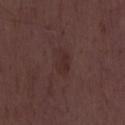Clinical impression:
Captured during whole-body skin photography for melanoma surveillance; the lesion was not biopsied.
Background:
Cropped from a total-body skin-imaging series; the visible field is about 15 mm. Automated image analysis of the tile measured an area of roughly 5 mm², an outline eccentricity of about 0.85 (0 = round, 1 = elongated), and a shape-asymmetry score of about 0.25 (0 = symmetric). It also reported a lesion color around L≈28 a*≈18 b*≈18 in CIELAB and roughly 5 lightness units darker than nearby skin. And it measured a border-irregularity index near 2.5/10, internal color variation of about 2 on a 0–10 scale, and a peripheral color-asymmetry measure near 0.5. The lesion's longest dimension is about 3.5 mm. This is a white-light tile. A male subject, in their 50s.